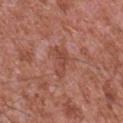biopsy status — catalogued during a skin exam; not biopsied | lesion diameter — ≈4 mm | lighting — white-light illumination | patient — male, aged approximately 45 | site — the chest | imaging modality — ~15 mm tile from a whole-body skin photo.